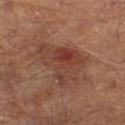The recorded lesion diameter is about 6.5 mm. A male patient aged around 65. The tile uses cross-polarized illumination. The lesion-visualizer software estimated internal color variation of about 6 on a 0–10 scale. A 15 mm close-up tile from a total-body photography series done for melanoma screening. The lesion is on the right lower leg.